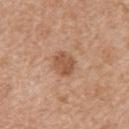Part of a total-body skin-imaging series; this lesion was reviewed on a skin check and was not flagged for biopsy. Imaged with white-light lighting. The recorded lesion diameter is about 3 mm. The lesion is located on the upper back. The lesion-visualizer software estimated a color-variation rating of about 2.5/10 and a peripheral color-asymmetry measure near 1. And it measured a detector confidence of about 100 out of 100 that the crop contains a lesion. A 15 mm crop from a total-body photograph taken for skin-cancer surveillance. The patient is a female roughly 55 years of age.